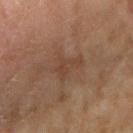biopsy status = catalogued during a skin exam; not biopsied | anatomic site = the right upper arm | image-analysis metrics = an area of roughly 4 mm², a shape eccentricity near 0.8, and two-axis asymmetry of about 0.65; an automated nevus-likeness rating near 0 out of 100 | lighting = cross-polarized | lesion size = ~3.5 mm (longest diameter) | imaging modality = ~15 mm tile from a whole-body skin photo | subject = female, aged approximately 60.